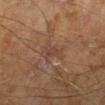biopsy status — catalogued during a skin exam; not biopsied | lighting — cross-polarized | subject — male, in their mid-60s | size — ~4 mm (longest diameter) | imaging modality — ~15 mm crop, total-body skin-cancer survey.